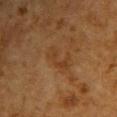Q: What did automated image analysis measure?
A: an area of roughly 5 mm², a shape eccentricity near 0.9, and a shape-asymmetry score of about 0.5 (0 = symmetric); an average lesion color of about L≈32 a*≈17 b*≈30 (CIELAB) and a lesion-to-skin contrast of about 5 (normalized; higher = more distinct); a within-lesion color-variation index near 2/10 and peripheral color asymmetry of about 0.5
Q: What lighting was used for the tile?
A: cross-polarized
Q: What is the lesion's diameter?
A: ~4 mm (longest diameter)
Q: What is the anatomic site?
A: the chest
Q: How was this image acquired?
A: ~15 mm crop, total-body skin-cancer survey
Q: What are the patient's age and sex?
A: male, aged 58–62